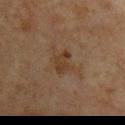image-analysis metrics: a footprint of about 5.5 mm², an outline eccentricity of about 0.55 (0 = round, 1 = elongated), and a symmetry-axis asymmetry near 0.3
location: the upper back
image: total-body-photography crop, ~15 mm field of view
lesion size: ≈2.5 mm
subject: male, about 65 years old